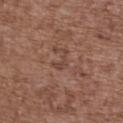workup — imaged on a skin check; not biopsied
subject — female, in their mid- to late 70s
image — ~15 mm crop, total-body skin-cancer survey
automated lesion analysis — an area of roughly 3 mm², a shape eccentricity near 0.85, and a shape-asymmetry score of about 0.75 (0 = symmetric); border irregularity of about 8.5 on a 0–10 scale, a within-lesion color-variation index near 0/10, and a peripheral color-asymmetry measure near 0; a nevus-likeness score of about 0/100
location — the upper back
illumination — white-light
lesion diameter — about 2.5 mm